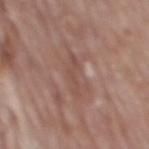This lesion was catalogued during total-body skin photography and was not selected for biopsy. The lesion is on the mid back. Approximately 4 mm at its widest. A male patient in their 70s. This image is a 15 mm lesion crop taken from a total-body photograph. Automated tile analysis of the lesion measured a shape-asymmetry score of about 0.5 (0 = symmetric). It also reported an average lesion color of about L≈49 a*≈20 b*≈24 (CIELAB), roughly 6 lightness units darker than nearby skin, and a normalized border contrast of about 5. The analysis additionally found a within-lesion color-variation index near 0/10.